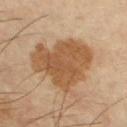Clinical impression: The lesion was tiled from a total-body skin photograph and was not biopsied. Context: Cropped from a total-body skin-imaging series; the visible field is about 15 mm. Located on the left upper arm. A male patient aged 58 to 62.